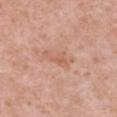Assessment:
The lesion was photographed on a routine skin check and not biopsied; there is no pathology result.
Context:
Automated tile analysis of the lesion measured a mean CIELAB color near L≈61 a*≈23 b*≈31, a lesion–skin lightness drop of about 7, and a lesion-to-skin contrast of about 5 (normalized; higher = more distinct). On the front of the torso. This image is a 15 mm lesion crop taken from a total-body photograph. A female patient about 40 years old. Captured under white-light illumination. Longest diameter approximately 4 mm.